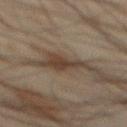– body site: the abdomen
– lesion size: about 5.5 mm
– subject: male, in their mid-40s
– tile lighting: cross-polarized illumination
– automated lesion analysis: a border-irregularity rating of about 5.5/10
– image: total-body-photography crop, ~15 mm field of view